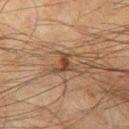lesion_size:
  long_diameter_mm_approx: 3.0
site: left thigh
patient:
  sex: male
  age_approx: 50
lighting: cross-polarized
automated_metrics:
  area_mm2_approx: 4.0
  eccentricity: 0.75
  shape_asymmetry: 0.55
  cielab_L: 35
  cielab_a: 16
  cielab_b: 27
  vs_skin_darker_L: 9.0
  vs_skin_contrast_norm: 8.0
  nevus_likeness_0_100: 50
  lesion_detection_confidence_0_100: 100
image:
  source: total-body photography crop
  field_of_view_mm: 15